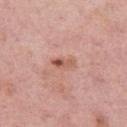<case>
<automated_metrics>
  <cielab_L>57</cielab_L>
  <cielab_a>24</cielab_a>
  <cielab_b>29</cielab_b>
  <vs_skin_darker_L>10.0</vs_skin_darker_L>
  <vs_skin_contrast_norm>7.0</vs_skin_contrast_norm>
  <border_irregularity_0_10>3.5</border_irregularity_0_10>
  <color_variation_0_10>6.5</color_variation_0_10>
  <peripheral_color_asymmetry>2.0</peripheral_color_asymmetry>
</automated_metrics>
<lesion_size>
  <long_diameter_mm_approx>3.0</long_diameter_mm_approx>
</lesion_size>
<lighting>white-light</lighting>
<site>left thigh</site>
<image>
  <source>total-body photography crop</source>
  <field_of_view_mm>15</field_of_view_mm>
</image>
<patient>
  <sex>female</sex>
  <age_approx>50</age_approx>
</patient>
</case>